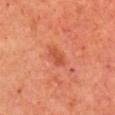The lesion was photographed on a routine skin check and not biopsied; there is no pathology result. The patient is a male aged 63–67. A 15 mm close-up tile from a total-body photography series done for melanoma screening. From the right upper arm.